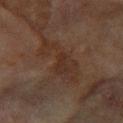Q: Is there a histopathology result?
A: imaged on a skin check; not biopsied
Q: Lesion location?
A: the right forearm
Q: What is the imaging modality?
A: 15 mm crop, total-body photography
Q: Who is the patient?
A: female, about 80 years old